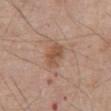workup: catalogued during a skin exam; not biopsied
patient: male, aged 78–82
image source: ~15 mm tile from a whole-body skin photo
body site: the abdomen
size: about 3 mm
illumination: white-light illumination